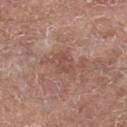Imaged during a routine full-body skin examination; the lesion was not biopsied and no histopathology is available.
Approximately 2.5 mm at its widest.
The subject is a male aged 63 to 67.
Automated image analysis of the tile measured a lesion color around L≈49 a*≈21 b*≈25 in CIELAB, roughly 7 lightness units darker than nearby skin, and a normalized lesion–skin contrast near 5. It also reported a nevus-likeness score of about 0/100 and a lesion-detection confidence of about 100/100.
This is a white-light tile.
From the left thigh.
Cropped from a whole-body photographic skin survey; the tile spans about 15 mm.